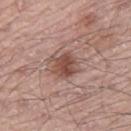A roughly 15 mm field-of-view crop from a total-body skin photograph.
This is a white-light tile.
The recorded lesion diameter is about 3.5 mm.
From the left leg.
Automated tile analysis of the lesion measured a lesion area of about 8 mm², a shape eccentricity near 0.5, and two-axis asymmetry of about 0.2. The software also gave an average lesion color of about L≈49 a*≈21 b*≈25 (CIELAB), about 11 CIELAB-L* units darker than the surrounding skin, and a normalized lesion–skin contrast near 8.5. And it measured a color-variation rating of about 4.5/10 and radial color variation of about 1.5.
A male subject about 70 years old.